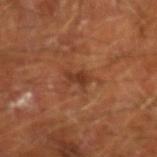Findings:
- notes: total-body-photography surveillance lesion; no biopsy
- size: ~3 mm (longest diameter)
- illumination: cross-polarized
- anatomic site: the left forearm
- imaging modality: ~15 mm tile from a whole-body skin photo
- subject: male, about 65 years old